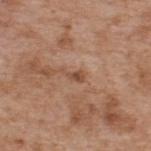Part of a total-body skin-imaging series; this lesion was reviewed on a skin check and was not flagged for biopsy. The lesion is located on the upper back. A male patient approximately 65 years of age. The recorded lesion diameter is about 2.5 mm. Cropped from a total-body skin-imaging series; the visible field is about 15 mm.